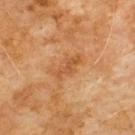| key | value |
|---|---|
| notes | total-body-photography surveillance lesion; no biopsy |
| imaging modality | 15 mm crop, total-body photography |
| body site | the chest |
| lighting | cross-polarized |
| subject | male, about 60 years old |